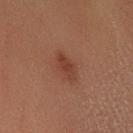{"biopsy_status": "not biopsied; imaged during a skin examination", "patient": {"sex": "female", "age_approx": 40}, "image": {"source": "total-body photography crop", "field_of_view_mm": 15}, "site": "left lower leg"}